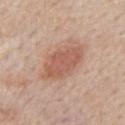Captured during whole-body skin photography for melanoma surveillance; the lesion was not biopsied.
A region of skin cropped from a whole-body photographic capture, roughly 15 mm wide.
Imaged with white-light lighting.
A male patient, aged approximately 60.
Approximately 6.5 mm at its widest.
Located on the mid back.